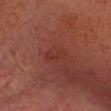Impression: Recorded during total-body skin imaging; not selected for excision or biopsy. Acquisition and patient details: The lesion is on the head or neck. This image is a 15 mm lesion crop taken from a total-body photograph. A male patient, approximately 60 years of age.